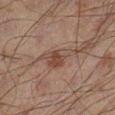Q: Was a biopsy performed?
A: no biopsy performed (imaged during a skin exam)
Q: What is the imaging modality?
A: 15 mm crop, total-body photography
Q: Illumination type?
A: cross-polarized illumination
Q: How large is the lesion?
A: ~5 mm (longest diameter)
Q: Where on the body is the lesion?
A: the left leg
Q: Who is the patient?
A: male, aged 58–62